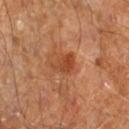| field | value |
|---|---|
| notes | no biopsy performed (imaged during a skin exam) |
| patient | male, aged approximately 60 |
| illumination | cross-polarized illumination |
| location | the right leg |
| image | total-body-photography crop, ~15 mm field of view |
| diameter | ~3 mm (longest diameter) |